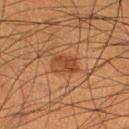follow-up: catalogued during a skin exam; not biopsied
TBP lesion metrics: a lesion color around L≈36 a*≈21 b*≈30 in CIELAB, a lesion–skin lightness drop of about 7, and a normalized border contrast of about 7; a border-irregularity rating of about 2.5/10 and a within-lesion color-variation index near 3.5/10
site: the right lower leg
imaging modality: ~15 mm crop, total-body skin-cancer survey
tile lighting: cross-polarized illumination
diameter: ≈3.5 mm
patient: male, aged approximately 50